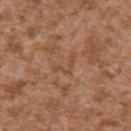{
  "image": {
    "source": "total-body photography crop",
    "field_of_view_mm": 15
  },
  "lesion_size": {
    "long_diameter_mm_approx": 3.5
  },
  "patient": {
    "sex": "male",
    "age_approx": 45
  },
  "site": "right upper arm",
  "lighting": "white-light",
  "automated_metrics": {
    "cielab_L": 48,
    "cielab_a": 21,
    "cielab_b": 32,
    "border_irregularity_0_10": 9.0
  }
}